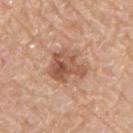Context:
A male patient about 60 years old. A region of skin cropped from a whole-body photographic capture, roughly 15 mm wide. On the chest. Captured under white-light illumination. Longest diameter approximately 4.5 mm.